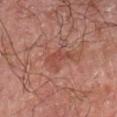| field | value |
|---|---|
| notes | no biopsy performed (imaged during a skin exam) |
| subject | male, aged 58 to 62 |
| image-analysis metrics | a lesion area of about 4.5 mm² and a symmetry-axis asymmetry near 0.4; a border-irregularity rating of about 5/10, internal color variation of about 0.5 on a 0–10 scale, and a peripheral color-asymmetry measure near 0 |
| acquisition | ~15 mm crop, total-body skin-cancer survey |
| site | the left forearm |